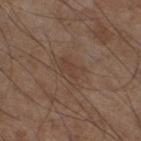Q: Was this lesion biopsied?
A: imaged on a skin check; not biopsied
Q: How was this image acquired?
A: ~15 mm tile from a whole-body skin photo
Q: Lesion location?
A: the left lower leg
Q: Patient demographics?
A: male, roughly 55 years of age
Q: How was the tile lit?
A: white-light
Q: How large is the lesion?
A: about 3.5 mm
Q: Automated lesion metrics?
A: a lesion area of about 6 mm² and two-axis asymmetry of about 0.3; a lesion color around L≈40 a*≈16 b*≈25 in CIELAB and roughly 5 lightness units darker than nearby skin; a border-irregularity index near 4/10, a within-lesion color-variation index near 2/10, and peripheral color asymmetry of about 0.5; a classifier nevus-likeness of about 0/100 and a detector confidence of about 100 out of 100 that the crop contains a lesion